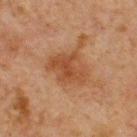Q: Was this lesion biopsied?
A: imaged on a skin check; not biopsied
Q: Lesion size?
A: ~5 mm (longest diameter)
Q: What is the anatomic site?
A: the front of the torso
Q: Who is the patient?
A: male, roughly 65 years of age
Q: What kind of image is this?
A: 15 mm crop, total-body photography
Q: Illumination type?
A: cross-polarized
Q: What did automated image analysis measure?
A: a lesion color around L≈38 a*≈19 b*≈30 in CIELAB, about 8 CIELAB-L* units darker than the surrounding skin, and a lesion-to-skin contrast of about 7 (normalized; higher = more distinct); an automated nevus-likeness rating near 10 out of 100